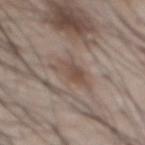{"lesion_size": {"long_diameter_mm_approx": 4.0}, "lighting": "white-light", "image": {"source": "total-body photography crop", "field_of_view_mm": 15}, "site": "chest", "patient": {"sex": "male", "age_approx": 55}}